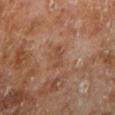Q: Was a biopsy performed?
A: total-body-photography surveillance lesion; no biopsy
Q: What is the imaging modality?
A: ~15 mm tile from a whole-body skin photo
Q: Where on the body is the lesion?
A: the left lower leg
Q: How was the tile lit?
A: cross-polarized illumination
Q: What are the patient's age and sex?
A: male, aged approximately 70